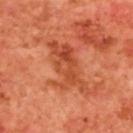A female patient, roughly 40 years of age. A 15 mm close-up tile from a total-body photography series done for melanoma screening. Located on the upper back. Longest diameter approximately 7 mm. Imaged with cross-polarized lighting.